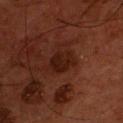Captured during whole-body skin photography for melanoma surveillance; the lesion was not biopsied.
This is a cross-polarized tile.
The subject is a male roughly 55 years of age.
The lesion is on the chest.
Cropped from a whole-body photographic skin survey; the tile spans about 15 mm.
The lesion's longest dimension is about 3.5 mm.
The lesion-visualizer software estimated an automated nevus-likeness rating near 0 out of 100 and lesion-presence confidence of about 100/100.